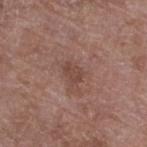Clinical impression:
Part of a total-body skin-imaging series; this lesion was reviewed on a skin check and was not flagged for biopsy.
Background:
From the leg. An algorithmic analysis of the crop reported a lesion area of about 4 mm², an outline eccentricity of about 0.7 (0 = round, 1 = elongated), and two-axis asymmetry of about 0.3. It also reported a mean CIELAB color near L≈44 a*≈19 b*≈24, a lesion–skin lightness drop of about 8, and a lesion-to-skin contrast of about 6 (normalized; higher = more distinct). And it measured a border-irregularity rating of about 3/10 and a within-lesion color-variation index near 1.5/10. A lesion tile, about 15 mm wide, cut from a 3D total-body photograph. A female patient, aged approximately 70. Imaged with white-light lighting. Measured at roughly 2.5 mm in maximum diameter.